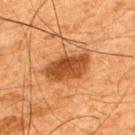The lesion was photographed on a routine skin check and not biopsied; there is no pathology result. Automated image analysis of the tile measured a lesion area of about 12 mm² and a symmetry-axis asymmetry near 0.2. It also reported radial color variation of about 1.5. It also reported an automated nevus-likeness rating near 90 out of 100 and a lesion-detection confidence of about 100/100. This is a cross-polarized tile. Longest diameter approximately 5.5 mm. A male subject, in their mid-60s. Located on the upper back. A region of skin cropped from a whole-body photographic capture, roughly 15 mm wide.